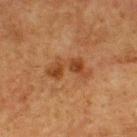biopsy status = total-body-photography surveillance lesion; no biopsy
size = ≈5 mm
subject = male, aged approximately 75
site = the upper back
imaging modality = total-body-photography crop, ~15 mm field of view
illumination = cross-polarized illumination